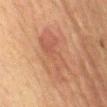Q: Was a biopsy performed?
A: total-body-photography surveillance lesion; no biopsy
Q: What did automated image analysis measure?
A: a lesion area of about 14 mm² and two-axis asymmetry of about 0.45; a lesion color around L≈45 a*≈20 b*≈27 in CIELAB, roughly 6 lightness units darker than nearby skin, and a normalized lesion–skin contrast near 5; a border-irregularity rating of about 5.5/10 and a color-variation rating of about 3/10
Q: Patient demographics?
A: female, approximately 60 years of age
Q: What lighting was used for the tile?
A: cross-polarized illumination
Q: What kind of image is this?
A: 15 mm crop, total-body photography
Q: Where on the body is the lesion?
A: the chest